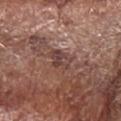The lesion was photographed on a routine skin check and not biopsied; there is no pathology result.
A male subject roughly 70 years of age.
The lesion is located on the right forearm.
A region of skin cropped from a whole-body photographic capture, roughly 15 mm wide.